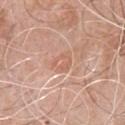Impression: This lesion was catalogued during total-body skin photography and was not selected for biopsy. Clinical summary: The total-body-photography lesion software estimated a lesion area of about 5.5 mm² and an eccentricity of roughly 0.65. The software also gave a classifier nevus-likeness of about 0/100. The tile uses white-light illumination. The subject is a male aged 68 to 72. A 15 mm crop from a total-body photograph taken for skin-cancer surveillance. The recorded lesion diameter is about 3 mm. Located on the chest.